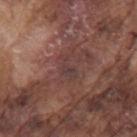Part of a total-body skin-imaging series; this lesion was reviewed on a skin check and was not flagged for biopsy.
From the left upper arm.
A lesion tile, about 15 mm wide, cut from a 3D total-body photograph.
A male subject about 75 years old.